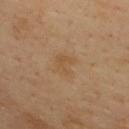• follow-up — no biopsy performed (imaged during a skin exam)
• tile lighting — cross-polarized illumination
• subject — male, aged 38 to 42
• size — ~2.5 mm (longest diameter)
• automated lesion analysis — a lesion area of about 5.5 mm² and two-axis asymmetry of about 0.3; an average lesion color of about L≈52 a*≈17 b*≈35 (CIELAB); a detector confidence of about 100 out of 100 that the crop contains a lesion
• imaging modality — ~15 mm crop, total-body skin-cancer survey
• body site — the upper back